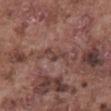Findings:
– workup — total-body-photography surveillance lesion; no biopsy
– subject — male, roughly 75 years of age
– acquisition — total-body-photography crop, ~15 mm field of view
– illumination — white-light
– image-analysis metrics — an average lesion color of about L≈41 a*≈19 b*≈22 (CIELAB), about 7 CIELAB-L* units darker than the surrounding skin, and a normalized lesion–skin contrast near 6; a nevus-likeness score of about 0/100 and a detector confidence of about 70 out of 100 that the crop contains a lesion
– lesion diameter — about 3 mm
– anatomic site — the abdomen